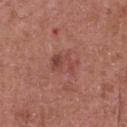Imaged during a routine full-body skin examination; the lesion was not biopsied and no histopathology is available.
From the chest.
Longest diameter approximately 3.5 mm.
A lesion tile, about 15 mm wide, cut from a 3D total-body photograph.
The tile uses white-light illumination.
A male patient aged 63–67.